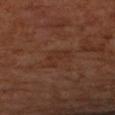This lesion was catalogued during total-body skin photography and was not selected for biopsy. The lesion-visualizer software estimated a lesion color around L≈30 a*≈20 b*≈26 in CIELAB, a lesion–skin lightness drop of about 4, and a normalized lesion–skin contrast near 4.5. And it measured a nevus-likeness score of about 0/100 and lesion-presence confidence of about 100/100. The patient is a female aged 63–67. Captured under cross-polarized illumination. The lesion's longest dimension is about 3.5 mm. A 15 mm close-up extracted from a 3D total-body photography capture. Located on the left forearm.